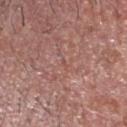Captured during whole-body skin photography for melanoma surveillance; the lesion was not biopsied.
This is a white-light tile.
A 15 mm crop from a total-body photograph taken for skin-cancer surveillance.
From the head or neck.
Measured at roughly 1 mm in maximum diameter.
The total-body-photography lesion software estimated a shape eccentricity near 0.8 and a symmetry-axis asymmetry near 0.45. And it measured an average lesion color of about L≈50 a*≈23 b*≈24 (CIELAB), roughly 5 lightness units darker than nearby skin, and a normalized border contrast of about 4.
The patient is a male in their mid- to late 40s.